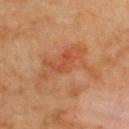Impression: Captured during whole-body skin photography for melanoma surveillance; the lesion was not biopsied. Image and clinical context: Located on the upper back. A lesion tile, about 15 mm wide, cut from a 3D total-body photograph. The lesion-visualizer software estimated an area of roughly 17 mm², a shape eccentricity near 0.85, and a shape-asymmetry score of about 0.5 (0 = symmetric). The analysis additionally found a lesion color around L≈54 a*≈26 b*≈38 in CIELAB, about 8 CIELAB-L* units darker than the surrounding skin, and a lesion-to-skin contrast of about 5.5 (normalized; higher = more distinct). And it measured a classifier nevus-likeness of about 0/100 and a detector confidence of about 100 out of 100 that the crop contains a lesion. The tile uses cross-polarized illumination. Approximately 7.5 mm at its widest. A female patient, aged 58 to 62.